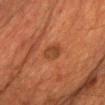Findings:
* workup · no biopsy performed (imaged during a skin exam)
* lesion size · ~2.5 mm (longest diameter)
* automated metrics · a footprint of about 4.5 mm², an outline eccentricity of about 0.65 (0 = round, 1 = elongated), and two-axis asymmetry of about 0.2
* subject · male, about 60 years old
* acquisition · total-body-photography crop, ~15 mm field of view
* illumination · cross-polarized illumination
* site · the chest